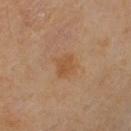Findings:
* follow-up · total-body-photography surveillance lesion; no biopsy
* anatomic site · the left upper arm
* size · ≈3 mm
* image source · ~15 mm tile from a whole-body skin photo
* illumination · cross-polarized
* patient · female, roughly 50 years of age
* automated lesion analysis · a lesion area of about 5 mm², an eccentricity of roughly 0.65, and a shape-asymmetry score of about 0.3 (0 = symmetric); roughly 6 lightness units darker than nearby skin and a normalized lesion–skin contrast near 6; a nevus-likeness score of about 5/100 and a detector confidence of about 100 out of 100 that the crop contains a lesion